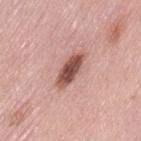workup — total-body-photography surveillance lesion; no biopsy | image — ~15 mm tile from a whole-body skin photo | anatomic site — the leg | patient — female, in their 50s.